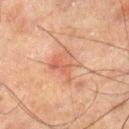| key | value |
|---|---|
| notes | no biopsy performed (imaged during a skin exam) |
| image source | ~15 mm tile from a whole-body skin photo |
| site | the right lower leg |
| subject | male, aged approximately 45 |